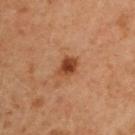Part of a total-body skin-imaging series; this lesion was reviewed on a skin check and was not flagged for biopsy. The lesion is located on the right upper arm. The recorded lesion diameter is about 2.5 mm. A male subject about 60 years old. This image is a 15 mm lesion crop taken from a total-body photograph. The lesion-visualizer software estimated a shape eccentricity near 0.65 and two-axis asymmetry of about 0.2. It also reported a mean CIELAB color near L≈33 a*≈20 b*≈28, a lesion–skin lightness drop of about 10, and a normalized border contrast of about 9.5. And it measured border irregularity of about 2 on a 0–10 scale, a within-lesion color-variation index near 2.5/10, and peripheral color asymmetry of about 0.5. The analysis additionally found an automated nevus-likeness rating near 95 out of 100 and lesion-presence confidence of about 100/100. Captured under cross-polarized illumination.